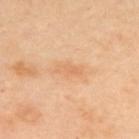  site: back
  lesion_size:
    long_diameter_mm_approx: 3.0
  patient:
    sex: female
    age_approx: 40
  image:
    source: total-body photography crop
    field_of_view_mm: 15
  automated_metrics:
    cielab_L: 70
    cielab_a: 22
    cielab_b: 40
    vs_skin_darker_L: 7.0
    vs_skin_contrast_norm: 5.0
    border_irregularity_0_10: 3.5
    color_variation_0_10: 1.0